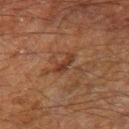Q: Was this lesion biopsied?
A: no biopsy performed (imaged during a skin exam)
Q: What kind of image is this?
A: 15 mm crop, total-body photography
Q: Lesion location?
A: the right thigh
Q: Who is the patient?
A: male, about 60 years old